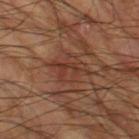| feature | finding |
|---|---|
| notes | imaged on a skin check; not biopsied |
| lesion diameter | ≈5 mm |
| subject | male, in their 60s |
| lighting | cross-polarized illumination |
| TBP lesion metrics | a shape eccentricity near 0.75; a border-irregularity rating of about 4/10, a within-lesion color-variation index near 4/10, and radial color variation of about 1.5; an automated nevus-likeness rating near 0 out of 100 and a detector confidence of about 80 out of 100 that the crop contains a lesion |
| acquisition | ~15 mm crop, total-body skin-cancer survey |
| body site | the left thigh |